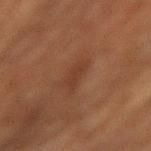biopsy_status: not biopsied; imaged during a skin examination
site: lower back
lesion_size:
  long_diameter_mm_approx: 3.0
patient:
  sex: male
  age_approx: 80
lighting: cross-polarized
image:
  source: total-body photography crop
  field_of_view_mm: 15